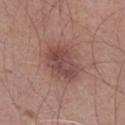Captured during whole-body skin photography for melanoma surveillance; the lesion was not biopsied. Captured under white-light illumination. A male subject, roughly 60 years of age. Cropped from a whole-body photographic skin survey; the tile spans about 15 mm. An algorithmic analysis of the crop reported a shape eccentricity near 0.65 and a shape-asymmetry score of about 0.2 (0 = symmetric). The software also gave an automated nevus-likeness rating near 20 out of 100 and lesion-presence confidence of about 100/100. The recorded lesion diameter is about 5 mm. On the right lower leg.